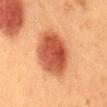Clinical impression: No biopsy was performed on this lesion — it was imaged during a full skin examination and was not determined to be concerning. Image and clinical context: The tile uses cross-polarized illumination. The lesion is on the mid back. The subject is a female in their 50s. The recorded lesion diameter is about 7 mm. A roughly 15 mm field-of-view crop from a total-body skin photograph.